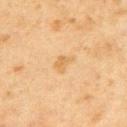Recorded during total-body skin imaging; not selected for excision or biopsy.
A male subject roughly 45 years of age.
The lesion is located on the upper back.
This image is a 15 mm lesion crop taken from a total-body photograph.
The lesion's longest dimension is about 3 mm.
The lesion-visualizer software estimated an area of roughly 3.5 mm² and an outline eccentricity of about 0.85 (0 = round, 1 = elongated). The software also gave internal color variation of about 1.5 on a 0–10 scale and peripheral color asymmetry of about 0.5. The software also gave an automated nevus-likeness rating near 0 out of 100 and lesion-presence confidence of about 100/100.
Imaged with cross-polarized lighting.